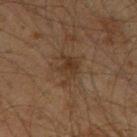Assessment: Captured during whole-body skin photography for melanoma surveillance; the lesion was not biopsied. Image and clinical context: Captured under cross-polarized illumination. A male patient aged 58–62. A close-up tile cropped from a whole-body skin photograph, about 15 mm across. Located on the left thigh.